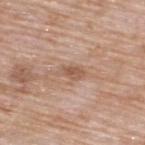Case summary:
- follow-up: catalogued during a skin exam; not biopsied
- diameter: about 3 mm
- image-analysis metrics: an average lesion color of about L≈56 a*≈19 b*≈30 (CIELAB), a lesion–skin lightness drop of about 9, and a normalized lesion–skin contrast near 6.5; a border-irregularity index near 3/10, a within-lesion color-variation index near 1.5/10, and peripheral color asymmetry of about 0.5
- imaging modality: ~15 mm crop, total-body skin-cancer survey
- site: the upper back
- subject: male, roughly 60 years of age